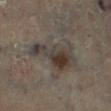Impression:
Captured during whole-body skin photography for melanoma surveillance; the lesion was not biopsied.
Image and clinical context:
A female subject about 60 years old. Measured at roughly 7 mm in maximum diameter. Automated image analysis of the tile measured a lesion area of about 17 mm² and an eccentricity of roughly 0.85. And it measured a mean CIELAB color near L≈36 a*≈8 b*≈17 and a normalized border contrast of about 8. The software also gave border irregularity of about 6.5 on a 0–10 scale and a peripheral color-asymmetry measure near 2. This image is a 15 mm lesion crop taken from a total-body photograph. The lesion is on the right lower leg. Captured under cross-polarized illumination.